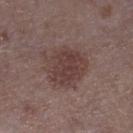| key | value |
|---|---|
| follow-up | imaged on a skin check; not biopsied |
| tile lighting | white-light |
| location | the right lower leg |
| image | ~15 mm crop, total-body skin-cancer survey |
| automated metrics | a footprint of about 17 mm², a shape eccentricity near 0.5, and a shape-asymmetry score of about 0.2 (0 = symmetric); an average lesion color of about L≈39 a*≈17 b*≈19 (CIELAB) and a lesion–skin lightness drop of about 8 |
| diameter | about 5.5 mm |
| patient | female, about 40 years old |